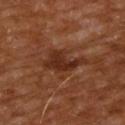Imaged during a routine full-body skin examination; the lesion was not biopsied and no histopathology is available.
A 15 mm close-up tile from a total-body photography series done for melanoma screening.
Measured at roughly 5 mm in maximum diameter.
Imaged with cross-polarized lighting.
Automated tile analysis of the lesion measured an area of roughly 8.5 mm², an outline eccentricity of about 0.8 (0 = round, 1 = elongated), and a symmetry-axis asymmetry near 0.35. And it measured an average lesion color of about L≈28 a*≈22 b*≈28 (CIELAB), about 9 CIELAB-L* units darker than the surrounding skin, and a normalized lesion–skin contrast near 8.5.
From the upper back.
A male subject in their mid- to late 60s.